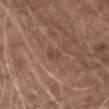Clinical impression: Recorded during total-body skin imaging; not selected for excision or biopsy. Background: The lesion is on the left forearm. Longest diameter approximately 2.5 mm. The tile uses white-light illumination. A male subject aged approximately 70. A 15 mm close-up tile from a total-body photography series done for melanoma screening.